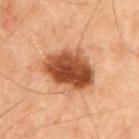Captured during whole-body skin photography for melanoma surveillance; the lesion was not biopsied. The tile uses cross-polarized illumination. The lesion is located on the front of the torso. Automated tile analysis of the lesion measured an area of roughly 20 mm², an eccentricity of roughly 0.75, and a shape-asymmetry score of about 0.15 (0 = symmetric). And it measured a border-irregularity index near 2/10 and internal color variation of about 6 on a 0–10 scale. It also reported an automated nevus-likeness rating near 95 out of 100 and lesion-presence confidence of about 100/100. A male subject, about 70 years old. This image is a 15 mm lesion crop taken from a total-body photograph.